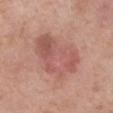biopsy status: imaged on a skin check; not biopsied | subject: male, about 60 years old | acquisition: ~15 mm tile from a whole-body skin photo | size: ≈6.5 mm | lighting: white-light | location: the arm.